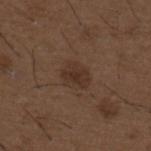Q: Is there a histopathology result?
A: catalogued during a skin exam; not biopsied
Q: Automated lesion metrics?
A: a mean CIELAB color near L≈32 a*≈16 b*≈24, roughly 7 lightness units darker than nearby skin, and a normalized lesion–skin contrast near 6.5; a nevus-likeness score of about 50/100 and a detector confidence of about 100 out of 100 that the crop contains a lesion
Q: What is the imaging modality?
A: total-body-photography crop, ~15 mm field of view
Q: What is the anatomic site?
A: the back
Q: Lesion size?
A: ≈3 mm
Q: Illumination type?
A: white-light illumination
Q: Who is the patient?
A: male, in their 50s